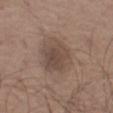| field | value |
|---|---|
| notes | catalogued during a skin exam; not biopsied |
| image source | 15 mm crop, total-body photography |
| lesion size | ~4.5 mm (longest diameter) |
| anatomic site | the left thigh |
| tile lighting | white-light |
| automated lesion analysis | an area of roughly 14 mm², a shape eccentricity near 0.3, and a symmetry-axis asymmetry near 0.2; a within-lesion color-variation index near 3/10 and radial color variation of about 1 |
| subject | male, approximately 75 years of age |